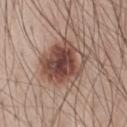Recorded during total-body skin imaging; not selected for excision or biopsy.
Imaged with white-light lighting.
About 5.5 mm across.
A 15 mm crop from a total-body photograph taken for skin-cancer surveillance.
The lesion is located on the chest.
Automated image analysis of the tile measured a lesion area of about 21 mm² and an eccentricity of roughly 0.5. It also reported an automated nevus-likeness rating near 85 out of 100 and lesion-presence confidence of about 100/100.
The subject is a male roughly 45 years of age.